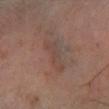biopsy status=total-body-photography surveillance lesion; no biopsy | anatomic site=the right lower leg | image source=15 mm crop, total-body photography | subject=male, approximately 70 years of age | lesion diameter=~5 mm (longest diameter) | tile lighting=cross-polarized.